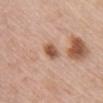No biopsy was performed on this lesion — it was imaged during a full skin examination and was not determined to be concerning. The subject is a female aged 48–52. Approximately 2.5 mm at its widest. On the upper back. A close-up tile cropped from a whole-body skin photograph, about 15 mm across. Captured under white-light illumination.